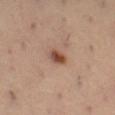{"biopsy_status": "not biopsied; imaged during a skin examination", "image": {"source": "total-body photography crop", "field_of_view_mm": 15}, "site": "left thigh", "patient": {"sex": "male", "age_approx": 55}}